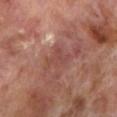Clinical impression:
This lesion was catalogued during total-body skin photography and was not selected for biopsy.
Image and clinical context:
This is a cross-polarized tile. A male patient, aged approximately 70. On the left lower leg. A lesion tile, about 15 mm wide, cut from a 3D total-body photograph. Longest diameter approximately 3.5 mm.